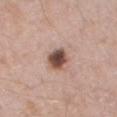Clinical impression: Recorded during total-body skin imaging; not selected for excision or biopsy. Context: A roughly 15 mm field-of-view crop from a total-body skin photograph. The patient is a male in their mid-60s. Imaged with white-light lighting. About 3 mm across. The lesion is on the left thigh.